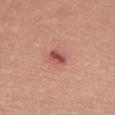| field | value |
|---|---|
| follow-up | total-body-photography surveillance lesion; no biopsy |
| acquisition | total-body-photography crop, ~15 mm field of view |
| location | the right thigh |
| tile lighting | white-light |
| patient | female, in their mid-50s |